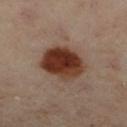notes — catalogued during a skin exam; not biopsied
acquisition — ~15 mm tile from a whole-body skin photo
lesion size — ~4.5 mm (longest diameter)
body site — the leg
illumination — cross-polarized
subject — female, aged around 60